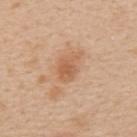Clinical impression: No biopsy was performed on this lesion — it was imaged during a full skin examination and was not determined to be concerning. Image and clinical context: An algorithmic analysis of the crop reported a footprint of about 5.5 mm², an eccentricity of roughly 0.75, and a symmetry-axis asymmetry near 0.3. It also reported a border-irregularity rating of about 3/10, a within-lesion color-variation index near 2.5/10, and peripheral color asymmetry of about 0.5. It also reported a nevus-likeness score of about 15/100 and a detector confidence of about 100 out of 100 that the crop contains a lesion. Longest diameter approximately 3 mm. A 15 mm close-up tile from a total-body photography series done for melanoma screening. Located on the upper back. A female subject, about 50 years old.